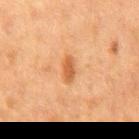{
  "biopsy_status": "not biopsied; imaged during a skin examination",
  "site": "mid back",
  "lesion_size": {
    "long_diameter_mm_approx": 3.0
  },
  "image": {
    "source": "total-body photography crop",
    "field_of_view_mm": 15
  },
  "automated_metrics": {
    "area_mm2_approx": 3.5,
    "eccentricity": 0.9,
    "shape_asymmetry": 0.25,
    "color_variation_0_10": 1.5,
    "peripheral_color_asymmetry": 0.5
  },
  "patient": {
    "sex": "male",
    "age_approx": 75
  },
  "lighting": "cross-polarized"
}